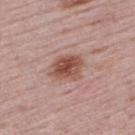A 15 mm crop from a total-body photograph taken for skin-cancer surveillance. The recorded lesion diameter is about 4 mm. Located on the upper back. A female subject, aged 48–52. Automated tile analysis of the lesion measured a footprint of about 10 mm², an outline eccentricity of about 0.65 (0 = round, 1 = elongated), and a shape-asymmetry score of about 0.2 (0 = symmetric). The software also gave a mean CIELAB color near L≈52 a*≈23 b*≈25, about 12 CIELAB-L* units darker than the surrounding skin, and a lesion-to-skin contrast of about 9 (normalized; higher = more distinct). It also reported a border-irregularity index near 2.5/10, a within-lesion color-variation index near 5/10, and a peripheral color-asymmetry measure near 1.5. This is a white-light tile.